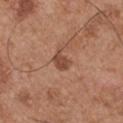workup: no biopsy performed (imaged during a skin exam) | location: the left upper arm | image source: ~15 mm crop, total-body skin-cancer survey | subject: male, aged 53 to 57 | tile lighting: white-light | automated metrics: a footprint of about 4 mm², a shape eccentricity near 0.7, and a shape-asymmetry score of about 0.3 (0 = symmetric); a border-irregularity index near 3/10; an automated nevus-likeness rating near 40 out of 100 and a detector confidence of about 100 out of 100 that the crop contains a lesion | lesion diameter: ~2.5 mm (longest diameter).